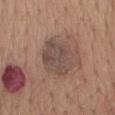| feature | finding |
|---|---|
| follow-up | imaged on a skin check; not biopsied |
| tile lighting | white-light illumination |
| anatomic site | the mid back |
| size | ≈4.5 mm |
| patient | female, approximately 65 years of age |
| imaging modality | ~15 mm tile from a whole-body skin photo |